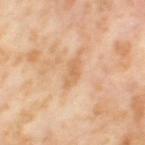Recorded during total-body skin imaging; not selected for excision or biopsy. Cropped from a whole-body photographic skin survey; the tile spans about 15 mm. An algorithmic analysis of the crop reported an eccentricity of roughly 0.9 and two-axis asymmetry of about 0.35. The analysis additionally found a border-irregularity index near 4/10, internal color variation of about 1 on a 0–10 scale, and radial color variation of about 0.5. The analysis additionally found a lesion-detection confidence of about 100/100. Longest diameter approximately 3.5 mm. This is a cross-polarized tile. A female patient, aged approximately 55. The lesion is located on the left thigh.